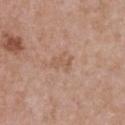Q: Was this lesion biopsied?
A: catalogued during a skin exam; not biopsied
Q: Where on the body is the lesion?
A: the chest
Q: What is the imaging modality?
A: ~15 mm tile from a whole-body skin photo
Q: How was the tile lit?
A: white-light
Q: What did automated image analysis measure?
A: a footprint of about 4.5 mm² and an outline eccentricity of about 0.65 (0 = round, 1 = elongated); a lesion color around L≈57 a*≈19 b*≈29 in CIELAB
Q: How large is the lesion?
A: ~3 mm (longest diameter)
Q: Patient demographics?
A: female, aged around 30